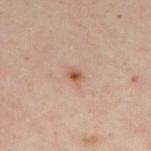patient — female, about 40 years old; image — ~15 mm crop, total-body skin-cancer survey; body site — the upper back; lesion diameter — about 1.5 mm.